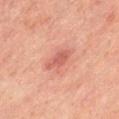{
  "biopsy_status": "not biopsied; imaged during a skin examination",
  "automated_metrics": {
    "area_mm2_approx": 4.0,
    "eccentricity": 0.8,
    "cielab_L": 61,
    "cielab_a": 32,
    "cielab_b": 31,
    "vs_skin_darker_L": 10.0,
    "vs_skin_contrast_norm": 6.5,
    "color_variation_0_10": 1.5,
    "peripheral_color_asymmetry": 0.5,
    "nevus_likeness_0_100": 5
  },
  "patient": {
    "sex": "female"
  },
  "site": "left thigh",
  "image": {
    "source": "total-body photography crop",
    "field_of_view_mm": 15
  },
  "lesion_size": {
    "long_diameter_mm_approx": 3.0
  },
  "lighting": "cross-polarized"
}